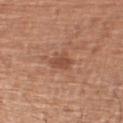The lesion's longest dimension is about 3 mm. From the right upper arm. A lesion tile, about 15 mm wide, cut from a 3D total-body photograph. The tile uses white-light illumination. A male subject, in their mid-60s.